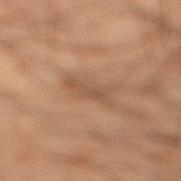Assessment:
Part of a total-body skin-imaging series; this lesion was reviewed on a skin check and was not flagged for biopsy.
Clinical summary:
Automated tile analysis of the lesion measured an area of roughly 6 mm², an eccentricity of roughly 0.85, and two-axis asymmetry of about 0.3. The analysis additionally found border irregularity of about 4 on a 0–10 scale, a color-variation rating of about 2/10, and a peripheral color-asymmetry measure near 0.5. It also reported a nevus-likeness score of about 0/100 and a detector confidence of about 95 out of 100 that the crop contains a lesion. A 15 mm crop from a total-body photograph taken for skin-cancer surveillance. The lesion is located on the right lower leg. The tile uses cross-polarized illumination. A male subject aged around 50.